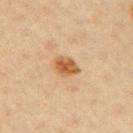No biopsy was performed on this lesion — it was imaged during a full skin examination and was not determined to be concerning. The lesion is on the right upper arm. A female subject aged around 45. Cropped from a total-body skin-imaging series; the visible field is about 15 mm.